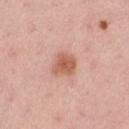Impression: Recorded during total-body skin imaging; not selected for excision or biopsy. Background: A female patient approximately 35 years of age. The tile uses white-light illumination. Located on the left thigh. A 15 mm close-up tile from a total-body photography series done for melanoma screening. Longest diameter approximately 3 mm. Automated tile analysis of the lesion measured an outline eccentricity of about 0.55 (0 = round, 1 = elongated) and a symmetry-axis asymmetry near 0.2. The software also gave internal color variation of about 2.5 on a 0–10 scale. And it measured a nevus-likeness score of about 90/100.